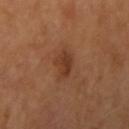This lesion was catalogued during total-body skin photography and was not selected for biopsy.
From the left arm.
The subject is a female in their 60s.
Cropped from a total-body skin-imaging series; the visible field is about 15 mm.